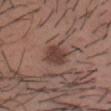Notes:
- subject · male, about 30 years old
- lighting · white-light illumination
- body site · the front of the torso
- automated metrics · a lesion area of about 6 mm², an outline eccentricity of about 0.45 (0 = round, 1 = elongated), and a symmetry-axis asymmetry near 0.2; an average lesion color of about L≈40 a*≈19 b*≈23 (CIELAB) and about 10 CIELAB-L* units darker than the surrounding skin; border irregularity of about 2 on a 0–10 scale and peripheral color asymmetry of about 1; an automated nevus-likeness rating near 60 out of 100 and a lesion-detection confidence of about 100/100
- acquisition · ~15 mm crop, total-body skin-cancer survey